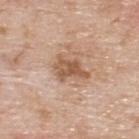Part of a total-body skin-imaging series; this lesion was reviewed on a skin check and was not flagged for biopsy.
From the upper back.
The recorded lesion diameter is about 4 mm.
The subject is a male in their 60s.
Captured under white-light illumination.
The total-body-photography lesion software estimated an average lesion color of about L≈55 a*≈20 b*≈32 (CIELAB) and a lesion-to-skin contrast of about 8 (normalized; higher = more distinct). The software also gave a border-irregularity index near 4.5/10, internal color variation of about 4.5 on a 0–10 scale, and peripheral color asymmetry of about 1.5.
A lesion tile, about 15 mm wide, cut from a 3D total-body photograph.